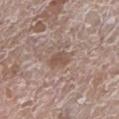{
  "lesion_size": {
    "long_diameter_mm_approx": 3.0
  },
  "patient": {
    "sex": "female",
    "age_approx": "85+"
  },
  "site": "left lower leg",
  "image": {
    "source": "total-body photography crop",
    "field_of_view_mm": 15
  },
  "automated_metrics": {
    "area_mm2_approx": 4.0,
    "shape_asymmetry": 0.3,
    "nevus_likeness_0_100": 35,
    "lesion_detection_confidence_0_100": 100
  },
  "lighting": "white-light"
}